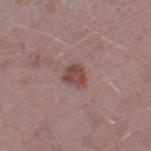{"biopsy_status": "not biopsied; imaged during a skin examination", "image": {"source": "total-body photography crop", "field_of_view_mm": 15}, "automated_metrics": {"eccentricity": 0.3, "cielab_L": 46, "cielab_a": 21, "cielab_b": 22, "vs_skin_darker_L": 10.0, "vs_skin_contrast_norm": 8.0, "color_variation_0_10": 2.0, "peripheral_color_asymmetry": 0.5}, "lesion_size": {"long_diameter_mm_approx": 2.5}, "patient": {"sex": "male", "age_approx": 40}, "site": "left thigh"}